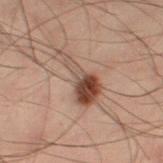No biopsy was performed on this lesion — it was imaged during a full skin examination and was not determined to be concerning.
The lesion's longest dimension is about 6.5 mm.
Captured under cross-polarized illumination.
This image is a 15 mm lesion crop taken from a total-body photograph.
The patient is a male aged 48–52.
From the left leg.